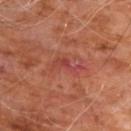Q: Is there a histopathology result?
A: imaged on a skin check; not biopsied
Q: Lesion location?
A: the front of the torso
Q: What are the patient's age and sex?
A: male, aged around 60
Q: What kind of image is this?
A: ~15 mm crop, total-body skin-cancer survey